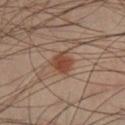<record>
<biopsy_status>not biopsied; imaged during a skin examination</biopsy_status>
<site>right lower leg</site>
<image>
  <source>total-body photography crop</source>
  <field_of_view_mm>15</field_of_view_mm>
</image>
<lesion_size>
  <long_diameter_mm_approx>2.5</long_diameter_mm_approx>
</lesion_size>
<patient>
  <sex>male</sex>
  <age_approx>40</age_approx>
</patient>
</record>